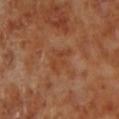Impression: Recorded during total-body skin imaging; not selected for excision or biopsy. Background: The patient is a male approximately 70 years of age. This is a cross-polarized tile. This image is a 15 mm lesion crop taken from a total-body photograph. The lesion is located on the left lower leg. The recorded lesion diameter is about 3.5 mm.